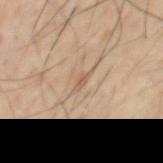{"image": {"source": "total-body photography crop", "field_of_view_mm": 15}, "site": "chest", "patient": {"sex": "male", "age_approx": 65}}